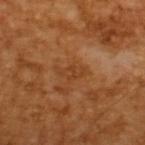Imaged during a routine full-body skin examination; the lesion was not biopsied and no histopathology is available. A lesion tile, about 15 mm wide, cut from a 3D total-body photograph. Approximately 2.5 mm at its widest. The patient is a male roughly 65 years of age. Captured under cross-polarized illumination.